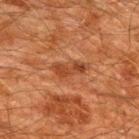Assessment: Imaged during a routine full-body skin examination; the lesion was not biopsied and no histopathology is available. Acquisition and patient details: Captured under cross-polarized illumination. Longest diameter approximately 4 mm. Automated image analysis of the tile measured a mean CIELAB color near L≈34 a*≈22 b*≈31 and a lesion-to-skin contrast of about 7 (normalized; higher = more distinct). And it measured a nevus-likeness score of about 0/100. On the upper back. A 15 mm close-up extracted from a 3D total-body photography capture. The subject is a male approximately 60 years of age.